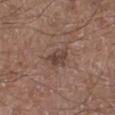Imaged during a routine full-body skin examination; the lesion was not biopsied and no histopathology is available.
A close-up tile cropped from a whole-body skin photograph, about 15 mm across.
The total-body-photography lesion software estimated an average lesion color of about L≈42 a*≈17 b*≈23 (CIELAB) and a lesion–skin lightness drop of about 9. It also reported a border-irregularity rating of about 3/10 and internal color variation of about 2.5 on a 0–10 scale. The software also gave a nevus-likeness score of about 0/100 and lesion-presence confidence of about 100/100.
The patient is a male roughly 55 years of age.
On the left lower leg.